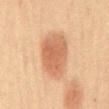This lesion was catalogued during total-body skin photography and was not selected for biopsy. Located on the mid back. The tile uses cross-polarized illumination. Approximately 6.5 mm at its widest. A close-up tile cropped from a whole-body skin photograph, about 15 mm across. A male subject aged approximately 60.